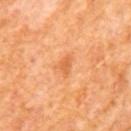Impression: No biopsy was performed on this lesion — it was imaged during a full skin examination and was not determined to be concerning. Clinical summary: The lesion is located on the mid back. A close-up tile cropped from a whole-body skin photograph, about 15 mm across. The tile uses cross-polarized illumination. Longest diameter approximately 2.5 mm. The patient is a male in their mid- to late 60s.